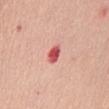Case summary:
* follow-up — imaged on a skin check; not biopsied
* patient — female, in their mid- to late 50s
* lighting — white-light
* anatomic site — the chest
* image source — total-body-photography crop, ~15 mm field of view
* lesion size — ~2.5 mm (longest diameter)
* image-analysis metrics — an area of roughly 4 mm², an outline eccentricity of about 0.8 (0 = round, 1 = elongated), and a shape-asymmetry score of about 0.3 (0 = symmetric); an average lesion color of about L≈57 a*≈38 b*≈27 (CIELAB) and a normalized border contrast of about 10; border irregularity of about 2.5 on a 0–10 scale and a peripheral color-asymmetry measure near 0.5; a classifier nevus-likeness of about 0/100 and a detector confidence of about 100 out of 100 that the crop contains a lesion